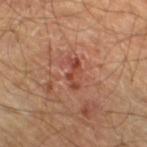Imaged during a routine full-body skin examination; the lesion was not biopsied and no histopathology is available. The lesion is located on the left lower leg. The subject is a male aged 53 to 57. Approximately 3.5 mm at its widest. A lesion tile, about 15 mm wide, cut from a 3D total-body photograph. Imaged with cross-polarized lighting.